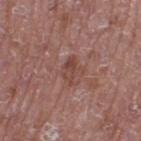Context: The tile uses white-light illumination. This image is a 15 mm lesion crop taken from a total-body photograph. A male patient, about 60 years old. Located on the right thigh.